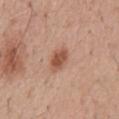Case summary:
- notes — imaged on a skin check; not biopsied
- lighting — white-light
- imaging modality — total-body-photography crop, ~15 mm field of view
- patient — male, aged 53–57
- size — ~3.5 mm (longest diameter)
- site — the mid back